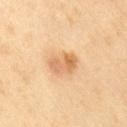notes — total-body-photography surveillance lesion; no biopsy | subject — female, roughly 60 years of age | site — the right thigh | acquisition — total-body-photography crop, ~15 mm field of view | lesion size — ≈3.5 mm | automated lesion analysis — a lesion area of about 7 mm², a shape eccentricity near 0.7, and a symmetry-axis asymmetry near 0.2; roughly 10 lightness units darker than nearby skin and a normalized border contrast of about 6.5; a nevus-likeness score of about 65/100 and a detector confidence of about 100 out of 100 that the crop contains a lesion.